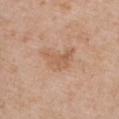Impression: The lesion was tiled from a total-body skin photograph and was not biopsied. Image and clinical context: A region of skin cropped from a whole-body photographic capture, roughly 15 mm wide. The lesion's longest dimension is about 4 mm. Automated tile analysis of the lesion measured a lesion-detection confidence of about 100/100. The tile uses white-light illumination. The subject is a female aged 38–42. From the chest.